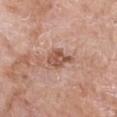| feature | finding |
|---|---|
| biopsy status | catalogued during a skin exam; not biopsied |
| image | ~15 mm tile from a whole-body skin photo |
| body site | the chest |
| image-analysis metrics | a lesion area of about 6 mm², an eccentricity of roughly 0.7, and two-axis asymmetry of about 0.3; internal color variation of about 3 on a 0–10 scale and radial color variation of about 1 |
| size | ≈3.5 mm |
| illumination | white-light |
| patient | female, in their mid-70s |